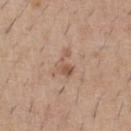biopsy_status: not biopsied; imaged during a skin examination
image:
  source: total-body photography crop
  field_of_view_mm: 15
patient:
  sex: male
  age_approx: 60
site: chest
automated_metrics:
  area_mm2_approx: 4.5
  eccentricity: 0.8
  shape_asymmetry: 0.65
  vs_skin_contrast_norm: 6.0
  border_irregularity_0_10: 7.5
  peripheral_color_asymmetry: 1.5
  nevus_likeness_0_100: 0
  lesion_detection_confidence_0_100: 100